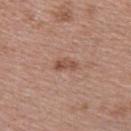<record>
<biopsy_status>not biopsied; imaged during a skin examination</biopsy_status>
<lighting>white-light</lighting>
<image>
  <source>total-body photography crop</source>
  <field_of_view_mm>15</field_of_view_mm>
</image>
<patient>
  <sex>female</sex>
  <age_approx>40</age_approx>
</patient>
<lesion_size>
  <long_diameter_mm_approx>3.0</long_diameter_mm_approx>
</lesion_size>
<site>upper back</site>
</record>